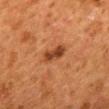biopsy status: total-body-photography surveillance lesion; no biopsy
location: the mid back
patient: female, aged around 50
image source: total-body-photography crop, ~15 mm field of view
automated lesion analysis: an area of roughly 5.5 mm² and an outline eccentricity of about 0.9 (0 = round, 1 = elongated); a mean CIELAB color near L≈36 a*≈23 b*≈32, roughly 10 lightness units darker than nearby skin, and a normalized border contrast of about 9; a border-irregularity index near 2/10, a within-lesion color-variation index near 2.5/10, and radial color variation of about 1
lighting: cross-polarized illumination